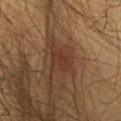location = the chest; patient = male, about 60 years old; illumination = cross-polarized; lesion size = ~2.5 mm (longest diameter); image source = ~15 mm tile from a whole-body skin photo.